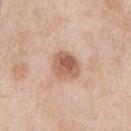This lesion was catalogued during total-body skin photography and was not selected for biopsy.
The tile uses white-light illumination.
Longest diameter approximately 3.5 mm.
Located on the left upper arm.
The subject is a male in their mid-50s.
A lesion tile, about 15 mm wide, cut from a 3D total-body photograph.
Automated image analysis of the tile measured a lesion area of about 8.5 mm² and an eccentricity of roughly 0.4. The software also gave a mean CIELAB color near L≈59 a*≈21 b*≈31 and roughly 13 lightness units darker than nearby skin.